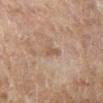<tbp_lesion>
  <biopsy_status>not biopsied; imaged during a skin examination</biopsy_status>
  <image>
    <source>total-body photography crop</source>
    <field_of_view_mm>15</field_of_view_mm>
  </image>
  <site>right lower leg</site>
  <patient>
    <sex>female</sex>
    <age_approx>60</age_approx>
  </patient>
</tbp_lesion>